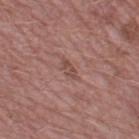Findings:
– notes: total-body-photography surveillance lesion; no biopsy